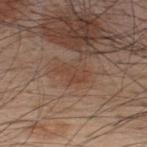– notes: catalogued during a skin exam; not biopsied
– image source: total-body-photography crop, ~15 mm field of view
– subject: male, approximately 35 years of age
– size: ≈4 mm
– location: the back